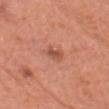notes: no biopsy performed (imaged during a skin exam) | patient: male, in their mid-50s | image source: ~15 mm crop, total-body skin-cancer survey | site: the head or neck.